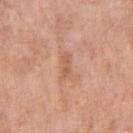  site: right upper arm
  image:
    source: total-body photography crop
    field_of_view_mm: 15
  lighting: white-light
  automated_metrics:
    eccentricity: 0.9
    shape_asymmetry: 0.2
    vs_skin_darker_L: 8.0
    vs_skin_contrast_norm: 5.5
    color_variation_0_10: 1.5
    peripheral_color_asymmetry: 0.5
  patient:
    sex: female
    age_approx: 70